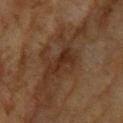Clinical impression: The lesion was tiled from a total-body skin photograph and was not biopsied. Background: Approximately 4 mm at its widest. A region of skin cropped from a whole-body photographic capture, roughly 15 mm wide. Imaged with cross-polarized lighting. The lesion is located on the head or neck. A female subject, roughly 60 years of age.